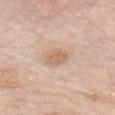Impression: Recorded during total-body skin imaging; not selected for excision or biopsy.